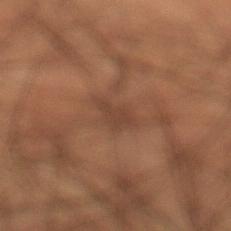The lesion is located on the left lower leg. A male patient approximately 50 years of age. A roughly 15 mm field-of-view crop from a total-body skin photograph.